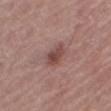Captured during whole-body skin photography for melanoma surveillance; the lesion was not biopsied.